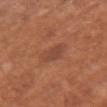diameter: ~2.5 mm (longest diameter); patient: female, in their mid-60s; illumination: white-light; automated metrics: a classifier nevus-likeness of about 5/100; site: the left arm; imaging modality: 15 mm crop, total-body photography.